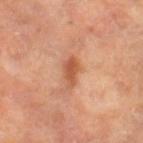workup = total-body-photography surveillance lesion; no biopsy | tile lighting = cross-polarized | patient = female, aged approximately 70 | image = 15 mm crop, total-body photography | location = the leg.